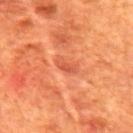  biopsy_status: not biopsied; imaged during a skin examination
  lesion_size:
    long_diameter_mm_approx: 3.0
  site: mid back
  patient:
    sex: male
    age_approx: 65
  automated_metrics:
    eccentricity: 0.85
    shape_asymmetry: 0.4
    cielab_L: 51
    cielab_a: 33
    cielab_b: 37
    vs_skin_darker_L: 8.0
    border_irregularity_0_10: 4.0
    peripheral_color_asymmetry: 0.0
    nevus_likeness_0_100: 0
  image:
    source: total-body photography crop
    field_of_view_mm: 15
  lighting: cross-polarized